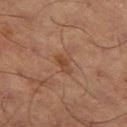{"biopsy_status": "not biopsied; imaged during a skin examination", "automated_metrics": {"border_irregularity_0_10": 3.5, "color_variation_0_10": 0.0}, "site": "right thigh", "lighting": "cross-polarized", "image": {"source": "total-body photography crop", "field_of_view_mm": 15}, "patient": {"sex": "male", "age_approx": 65}}